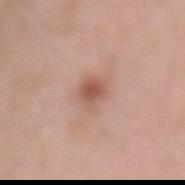Cropped from a whole-body photographic skin survey; the tile spans about 15 mm.
An algorithmic analysis of the crop reported a shape eccentricity near 0.5 and a symmetry-axis asymmetry near 0.2. The analysis additionally found a border-irregularity rating of about 2/10, a color-variation rating of about 3/10, and radial color variation of about 1.
Located on the mid back.
The recorded lesion diameter is about 3 mm.
Imaged with white-light lighting.
A female subject, aged around 70.